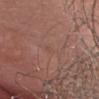Case summary:
* lighting — white-light illumination
* image source — ~15 mm tile from a whole-body skin photo
* diameter — about 1 mm
* anatomic site — the head or neck
* subject — male, roughly 50 years of age